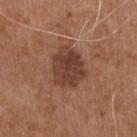| key | value |
|---|---|
| automated lesion analysis | an area of roughly 14 mm², a shape eccentricity near 0.6, and two-axis asymmetry of about 0.15; an average lesion color of about L≈40 a*≈22 b*≈27 (CIELAB) and a lesion–skin lightness drop of about 11; a within-lesion color-variation index near 3.5/10 and peripheral color asymmetry of about 1; an automated nevus-likeness rating near 60 out of 100 and a detector confidence of about 100 out of 100 that the crop contains a lesion |
| image | 15 mm crop, total-body photography |
| body site | the chest |
| diameter | about 5 mm |
| illumination | white-light |
| subject | male, aged 68 to 72 |